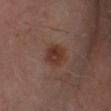Image and clinical context: Captured under cross-polarized illumination. Measured at roughly 3 mm in maximum diameter. From the right lower leg. A region of skin cropped from a whole-body photographic capture, roughly 15 mm wide. A male subject about 60 years old.